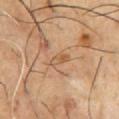Q: Was this lesion biopsied?
A: catalogued during a skin exam; not biopsied
Q: Illumination type?
A: cross-polarized illumination
Q: What did automated image analysis measure?
A: a lesion area of about 3 mm²; an automated nevus-likeness rating near 0 out of 100
Q: Lesion location?
A: the front of the torso
Q: How large is the lesion?
A: about 2.5 mm
Q: Who is the patient?
A: male, aged around 55
Q: What is the imaging modality?
A: 15 mm crop, total-body photography